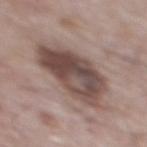A male patient aged approximately 65.
About 7 mm across.
Located on the upper back.
A 15 mm crop from a total-body photograph taken for skin-cancer surveillance.